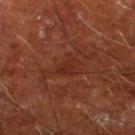Captured during whole-body skin photography for melanoma surveillance; the lesion was not biopsied.
A male patient about 65 years old.
A lesion tile, about 15 mm wide, cut from a 3D total-body photograph.
Automated tile analysis of the lesion measured a lesion area of about 3.5 mm², a shape eccentricity near 0.7, and a symmetry-axis asymmetry near 0.35. The software also gave an average lesion color of about L≈27 a*≈25 b*≈28 (CIELAB), a lesion–skin lightness drop of about 5, and a normalized lesion–skin contrast near 5.5. It also reported a border-irregularity index near 3.5/10 and a within-lesion color-variation index near 1/10.
Measured at roughly 2.5 mm in maximum diameter.
On the leg.